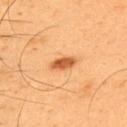Q: Is there a histopathology result?
A: catalogued during a skin exam; not biopsied
Q: Who is the patient?
A: male, in their mid-50s
Q: How was this image acquired?
A: total-body-photography crop, ~15 mm field of view
Q: Lesion size?
A: ~3 mm (longest diameter)
Q: What is the anatomic site?
A: the back
Q: How was the tile lit?
A: cross-polarized